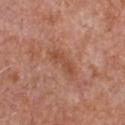Part of a total-body skin-imaging series; this lesion was reviewed on a skin check and was not flagged for biopsy. The subject is a male aged approximately 65. The total-body-photography lesion software estimated an average lesion color of about L≈49 a*≈25 b*≈32 (CIELAB) and a lesion–skin lightness drop of about 7. The lesion is located on the chest. A close-up tile cropped from a whole-body skin photograph, about 15 mm across.